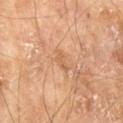image = 15 mm crop, total-body photography
patient = male, approximately 70 years of age
site = the leg